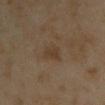Imaged during a routine full-body skin examination; the lesion was not biopsied and no histopathology is available. Automated image analysis of the tile measured an outline eccentricity of about 0.65 (0 = round, 1 = elongated). The analysis additionally found a border-irregularity rating of about 2/10, a color-variation rating of about 2.5/10, and a peripheral color-asymmetry measure near 1. Longest diameter approximately 2.5 mm. A female patient, in their mid- to late 30s. The lesion is located on the chest. The tile uses cross-polarized illumination. A roughly 15 mm field-of-view crop from a total-body skin photograph.